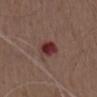{
  "biopsy_status": "not biopsied; imaged during a skin examination",
  "lesion_size": {
    "long_diameter_mm_approx": 3.5
  },
  "site": "chest",
  "image": {
    "source": "total-body photography crop",
    "field_of_view_mm": 15
  },
  "automated_metrics": {
    "cielab_L": 33,
    "cielab_a": 25,
    "cielab_b": 20,
    "vs_skin_darker_L": 13.0,
    "vs_skin_contrast_norm": 11.0,
    "peripheral_color_asymmetry": 1.5,
    "nevus_likeness_0_100": 0,
    "lesion_detection_confidence_0_100": 100
  },
  "patient": {
    "sex": "male",
    "age_approx": 70
  }
}